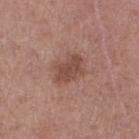workup — total-body-photography surveillance lesion; no biopsy | anatomic site — the left lower leg | subject — male, roughly 55 years of age | image-analysis metrics — a mean CIELAB color near L≈47 a*≈21 b*≈25 and a lesion-to-skin contrast of about 7 (normalized; higher = more distinct) | lesion size — ~4 mm (longest diameter) | tile lighting — white-light illumination | image — 15 mm crop, total-body photography.